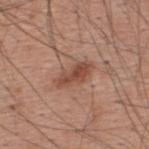workup=imaged on a skin check; not biopsied | patient=male, roughly 60 years of age | imaging modality=~15 mm crop, total-body skin-cancer survey | body site=the upper back | tile lighting=white-light illumination.